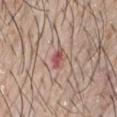The tile uses white-light illumination.
A male patient, in their mid-60s.
The lesion is located on the chest.
A close-up tile cropped from a whole-body skin photograph, about 15 mm across.
Longest diameter approximately 2.5 mm.